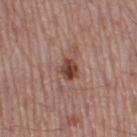Notes:
* workup · no biopsy performed (imaged during a skin exam)
* location · the right thigh
* tile lighting · white-light
* image source · total-body-photography crop, ~15 mm field of view
* subject · male, in their mid-50s